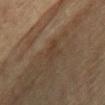* biopsy status: imaged on a skin check; not biopsied
* image: 15 mm crop, total-body photography
* lesion diameter: ≈3 mm
* subject: female, aged 78 to 82
* location: the chest